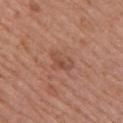Part of a total-body skin-imaging series; this lesion was reviewed on a skin check and was not flagged for biopsy. A 15 mm crop from a total-body photograph taken for skin-cancer surveillance. A female subject, about 50 years old. On the chest.